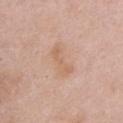Impression:
Imaged during a routine full-body skin examination; the lesion was not biopsied and no histopathology is available.
Clinical summary:
On the chest. A lesion tile, about 15 mm wide, cut from a 3D total-body photograph. The lesion's longest dimension is about 4 mm. The total-body-photography lesion software estimated a mean CIELAB color near L≈63 a*≈19 b*≈31, a lesion–skin lightness drop of about 6, and a lesion-to-skin contrast of about 5.5 (normalized; higher = more distinct). It also reported an automated nevus-likeness rating near 0 out of 100 and a lesion-detection confidence of about 100/100. The subject is a female roughly 60 years of age.